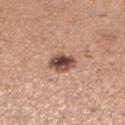{"biopsy_status": "not biopsied; imaged during a skin examination", "patient": {"sex": "female", "age_approx": 30}, "lesion_size": {"long_diameter_mm_approx": 3.0}, "lighting": "white-light", "image": {"source": "total-body photography crop", "field_of_view_mm": 15}, "site": "left lower leg"}